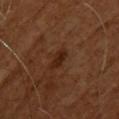This lesion was catalogued during total-body skin photography and was not selected for biopsy. A female subject, approximately 55 years of age. A lesion tile, about 15 mm wide, cut from a 3D total-body photograph. Automated tile analysis of the lesion measured a mean CIELAB color near L≈25 a*≈21 b*≈28 and about 8 CIELAB-L* units darker than the surrounding skin. The software also gave a lesion-detection confidence of about 100/100. On the upper back. The tile uses cross-polarized illumination. About 3 mm across.